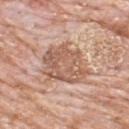This lesion was catalogued during total-body skin photography and was not selected for biopsy. The subject is a male roughly 80 years of age. Measured at roughly 6 mm in maximum diameter. Automated image analysis of the tile measured an area of roughly 20 mm², a shape eccentricity near 0.65, and two-axis asymmetry of about 0.25. The software also gave a classifier nevus-likeness of about 0/100 and a detector confidence of about 90 out of 100 that the crop contains a lesion. The tile uses white-light illumination. On the upper back. Cropped from a total-body skin-imaging series; the visible field is about 15 mm.